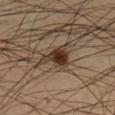Imaged during a routine full-body skin examination; the lesion was not biopsied and no histopathology is available. Captured under cross-polarized illumination. The patient is a male in their mid- to late 30s. The lesion's longest dimension is about 3.5 mm. The lesion is located on the right lower leg. An algorithmic analysis of the crop reported a lesion color around L≈30 a*≈14 b*≈23 in CIELAB, roughly 12 lightness units darker than nearby skin, and a lesion-to-skin contrast of about 11.5 (normalized; higher = more distinct). And it measured a nevus-likeness score of about 100/100 and a detector confidence of about 100 out of 100 that the crop contains a lesion. A lesion tile, about 15 mm wide, cut from a 3D total-body photograph.